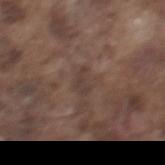workup=total-body-photography surveillance lesion; no biopsy